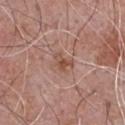| key | value |
|---|---|
| acquisition | ~15 mm crop, total-body skin-cancer survey |
| lighting | white-light illumination |
| anatomic site | the chest |
| subject | male, aged 68–72 |
| automated metrics | radial color variation of about 1; a nevus-likeness score of about 5/100 |